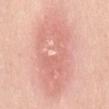  biopsy_status: not biopsied; imaged during a skin examination
  patient:
    sex: female
    age_approx: 45
  lighting: white-light
  image:
    source: total-body photography crop
    field_of_view_mm: 15
  site: mid back